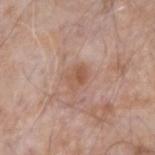Assessment: The lesion was photographed on a routine skin check and not biopsied; there is no pathology result. Context: A 15 mm close-up tile from a total-body photography series done for melanoma screening. The lesion-visualizer software estimated a lesion color around L≈54 a*≈21 b*≈30 in CIELAB, a lesion–skin lightness drop of about 8, and a normalized border contrast of about 6.5. It also reported a border-irregularity index near 2/10, internal color variation of about 3.5 on a 0–10 scale, and radial color variation of about 1. Imaged with white-light lighting. A male patient about 75 years old. From the right forearm. Longest diameter approximately 2.5 mm.